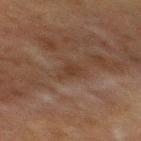This lesion was catalogued during total-body skin photography and was not selected for biopsy.
A region of skin cropped from a whole-body photographic capture, roughly 15 mm wide.
The patient is a male aged 73–77.
Imaged with cross-polarized lighting.
The lesion is on the mid back.